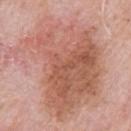follow-up: no biopsy performed (imaged during a skin exam) | location: the chest | patient: male, in their 80s | tile lighting: white-light illumination | image source: ~15 mm tile from a whole-body skin photo | automated metrics: an area of roughly 80 mm², an outline eccentricity of about 0.65 (0 = round, 1 = elongated), and two-axis asymmetry of about 0.35 | lesion diameter: ≈11.5 mm.